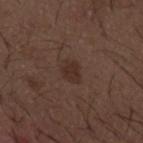Q: Is there a histopathology result?
A: catalogued during a skin exam; not biopsied
Q: What kind of image is this?
A: 15 mm crop, total-body photography
Q: Patient demographics?
A: male, approximately 50 years of age
Q: What did automated image analysis measure?
A: a footprint of about 5.5 mm², an outline eccentricity of about 0.75 (0 = round, 1 = elongated), and two-axis asymmetry of about 0.2; an average lesion color of about L≈30 a*≈15 b*≈21 (CIELAB) and a lesion–skin lightness drop of about 6; a border-irregularity rating of about 2/10, internal color variation of about 1 on a 0–10 scale, and peripheral color asymmetry of about 0.5
Q: What lighting was used for the tile?
A: white-light illumination
Q: Lesion location?
A: the mid back